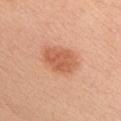About 4.5 mm across. A female patient, aged approximately 45. Automated tile analysis of the lesion measured a lesion area of about 12 mm², a shape eccentricity near 0.75, and a shape-asymmetry score of about 0.2 (0 = symmetric). The analysis additionally found a color-variation rating of about 2.5/10. And it measured a nevus-likeness score of about 95/100 and a detector confidence of about 100 out of 100 that the crop contains a lesion. A roughly 15 mm field-of-view crop from a total-body skin photograph. The lesion is located on the chest.